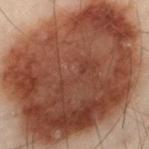Assessment:
Imaged during a routine full-body skin examination; the lesion was not biopsied and no histopathology is available.
Acquisition and patient details:
From the left thigh. A roughly 15 mm field-of-view crop from a total-body skin photograph. Measured at roughly 14 mm in maximum diameter. The tile uses cross-polarized illumination. A male subject, roughly 50 years of age.